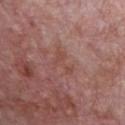biopsy status — total-body-photography surveillance lesion; no biopsy | illumination — white-light illumination | TBP lesion metrics — an area of roughly 4.5 mm², a shape eccentricity near 0.85, and a shape-asymmetry score of about 0.7 (0 = symmetric); a lesion–skin lightness drop of about 6 | anatomic site — the chest | lesion diameter — about 3.5 mm | image source — total-body-photography crop, ~15 mm field of view | subject — male, aged 68–72.